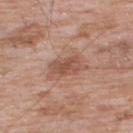Findings:
– biopsy status · total-body-photography surveillance lesion; no biopsy
– anatomic site · the upper back
– automated metrics · an average lesion color of about L≈52 a*≈21 b*≈28 (CIELAB) and a lesion–skin lightness drop of about 10
– lighting · white-light illumination
– image · total-body-photography crop, ~15 mm field of view
– subject · male, about 60 years old
– lesion size · ≈4 mm